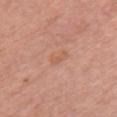  biopsy_status: not biopsied; imaged during a skin examination
  image:
    source: total-body photography crop
    field_of_view_mm: 15
  site: chest
  automated_metrics:
    cielab_L: 59
    cielab_a: 23
    cielab_b: 32
    vs_skin_darker_L: 5.0
    vs_skin_contrast_norm: 4.5
    peripheral_color_asymmetry: 0.0
  lighting: white-light
  patient:
    sex: female
    age_approx: 60
  lesion_size:
    long_diameter_mm_approx: 2.5